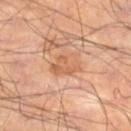The lesion was photographed on a routine skin check and not biopsied; there is no pathology result. The tile uses cross-polarized illumination. Located on the right thigh. A male patient, aged around 55. A region of skin cropped from a whole-body photographic capture, roughly 15 mm wide. About 3.5 mm across.